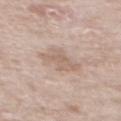Captured during whole-body skin photography for melanoma surveillance; the lesion was not biopsied. A lesion tile, about 15 mm wide, cut from a 3D total-body photograph. The subject is a male aged around 70. From the front of the torso.